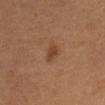<case>
  <lighting>cross-polarized</lighting>
  <site>abdomen</site>
  <patient>
    <sex>male</sex>
    <age_approx>60</age_approx>
  </patient>
  <lesion_size>
    <long_diameter_mm_approx>2.5</long_diameter_mm_approx>
  </lesion_size>
  <image>
    <source>total-body photography crop</source>
    <field_of_view_mm>15</field_of_view_mm>
  </image>
  <automated_metrics>
    <eccentricity>0.8</eccentricity>
    <shape_asymmetry>0.3</shape_asymmetry>
    <nevus_likeness_0_100>90</nevus_likeness_0_100>
    <lesion_detection_confidence_0_100>100</lesion_detection_confidence_0_100>
  </automated_metrics>
</case>